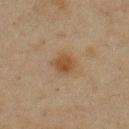The lesion's longest dimension is about 2.5 mm.
A 15 mm close-up tile from a total-body photography series done for melanoma screening.
Located on the chest.
A male subject, in their mid- to late 40s.